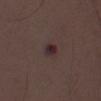Clinical impression:
No biopsy was performed on this lesion — it was imaged during a full skin examination and was not determined to be concerning.
Background:
A male subject, aged approximately 50. The lesion's longest dimension is about 3 mm. The tile uses white-light illumination. A 15 mm close-up tile from a total-body photography series done for melanoma screening.